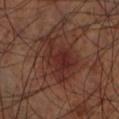Impression: The lesion was photographed on a routine skin check and not biopsied; there is no pathology result. Acquisition and patient details: From the left lower leg. A 15 mm close-up extracted from a 3D total-body photography capture. Captured under cross-polarized illumination. Longest diameter approximately 6 mm. The patient is a male roughly 70 years of age.